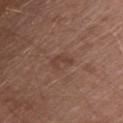follow-up — catalogued during a skin exam; not biopsied
body site — the upper back
image — total-body-photography crop, ~15 mm field of view
subject — female, aged around 50